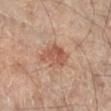Assessment: Part of a total-body skin-imaging series; this lesion was reviewed on a skin check and was not flagged for biopsy. Context: The lesion is on the right lower leg. Cropped from a total-body skin-imaging series; the visible field is about 15 mm. The total-body-photography lesion software estimated an outline eccentricity of about 0.7 (0 = round, 1 = elongated). It also reported a lesion color around L≈51 a*≈22 b*≈29 in CIELAB, a lesion–skin lightness drop of about 9, and a normalized lesion–skin contrast near 7. The analysis additionally found border irregularity of about 4 on a 0–10 scale, a color-variation rating of about 3/10, and peripheral color asymmetry of about 1. The analysis additionally found a nevus-likeness score of about 45/100 and a lesion-detection confidence of about 100/100. The subject is a male in their mid-60s. Longest diameter approximately 4 mm. Captured under cross-polarized illumination.